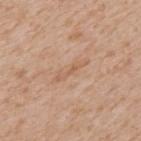Imaged during a routine full-body skin examination; the lesion was not biopsied and no histopathology is available.